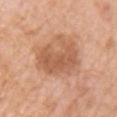The lesion was photographed on a routine skin check and not biopsied; there is no pathology result. The tile uses white-light illumination. The lesion-visualizer software estimated lesion-presence confidence of about 100/100. The recorded lesion diameter is about 6 mm. Located on the chest. A 15 mm crop from a total-body photograph taken for skin-cancer surveillance. A female patient in their mid- to late 50s.